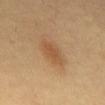Q: Was a biopsy performed?
A: imaged on a skin check; not biopsied
Q: What did automated image analysis measure?
A: an area of roughly 6 mm² and a shape-asymmetry score of about 0.2 (0 = symmetric); a mean CIELAB color near L≈54 a*≈20 b*≈38, a lesion–skin lightness drop of about 8, and a normalized lesion–skin contrast near 6; border irregularity of about 2 on a 0–10 scale and a within-lesion color-variation index near 1.5/10; a nevus-likeness score of about 80/100 and a lesion-detection confidence of about 100/100
Q: Who is the patient?
A: female, aged approximately 60
Q: What is the imaging modality?
A: 15 mm crop, total-body photography
Q: Where on the body is the lesion?
A: the mid back